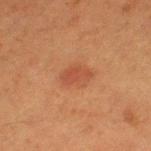Findings:
* workup — imaged on a skin check; not biopsied
* imaging modality — 15 mm crop, total-body photography
* anatomic site — the left thigh
* patient — female, in their 50s